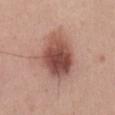Assessment: Captured during whole-body skin photography for melanoma surveillance; the lesion was not biopsied. Context: The subject is a male aged 48 to 52. On the mid back. About 6 mm across. A 15 mm close-up tile from a total-body photography series done for melanoma screening. Automated image analysis of the tile measured a classifier nevus-likeness of about 100/100 and a lesion-detection confidence of about 100/100.